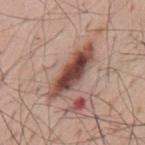  biopsy_status: not biopsied; imaged during a skin examination
  site: mid back
  patient:
    sex: male
    age_approx: 55
  image:
    source: total-body photography crop
    field_of_view_mm: 15
  automated_metrics:
    eccentricity: 0.95
    shape_asymmetry: 0.2
    vs_skin_darker_L: 16.0
    vs_skin_contrast_norm: 11.0
    color_variation_0_10: 8.0